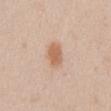* workup — catalogued during a skin exam; not biopsied
* anatomic site — the abdomen
* tile lighting — white-light
* diameter — about 3 mm
* automated metrics — an outline eccentricity of about 0.75 (0 = round, 1 = elongated) and a symmetry-axis asymmetry near 0.15; an average lesion color of about L≈62 a*≈21 b*≈33 (CIELAB) and a lesion–skin lightness drop of about 11
* subject — female, aged approximately 30
* image — ~15 mm crop, total-body skin-cancer survey